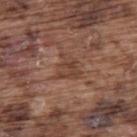| feature | finding |
|---|---|
| follow-up | imaged on a skin check; not biopsied |
| subject | male, roughly 75 years of age |
| image | ~15 mm tile from a whole-body skin photo |
| site | the back |
| diameter | ≈3 mm |
| lighting | white-light |
| automated lesion analysis | a border-irregularity rating of about 4.5/10, internal color variation of about 3.5 on a 0–10 scale, and a peripheral color-asymmetry measure near 1.5; an automated nevus-likeness rating near 0 out of 100 and a detector confidence of about 80 out of 100 that the crop contains a lesion |